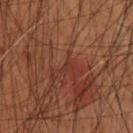Q: Was this lesion biopsied?
A: total-body-photography surveillance lesion; no biopsy
Q: What did automated image analysis measure?
A: an eccentricity of roughly 0.75 and a shape-asymmetry score of about 0.3 (0 = symmetric); roughly 4 lightness units darker than nearby skin and a normalized border contrast of about 4; a classifier nevus-likeness of about 0/100 and a lesion-detection confidence of about 5/100
Q: How large is the lesion?
A: ~1.5 mm (longest diameter)
Q: What is the imaging modality?
A: ~15 mm crop, total-body skin-cancer survey
Q: What is the anatomic site?
A: the upper back
Q: What lighting was used for the tile?
A: cross-polarized
Q: What are the patient's age and sex?
A: male, aged approximately 60